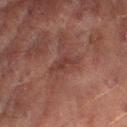Clinical impression:
Captured during whole-body skin photography for melanoma surveillance; the lesion was not biopsied.
Image and clinical context:
The recorded lesion diameter is about 4 mm. This image is a 15 mm lesion crop taken from a total-body photograph. The total-body-photography lesion software estimated a classifier nevus-likeness of about 0/100 and a lesion-detection confidence of about 65/100. Located on the right lower leg. Imaged with cross-polarized lighting. The patient is a male aged around 70.